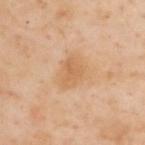notes: total-body-photography surveillance lesion; no biopsy
image: ~15 mm crop, total-body skin-cancer survey
patient: male, aged approximately 55
location: the back